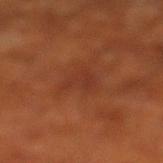The lesion was tiled from a total-body skin photograph and was not biopsied.
Longest diameter approximately 3 mm.
The lesion is on the left lower leg.
A male subject, approximately 70 years of age.
This image is a 15 mm lesion crop taken from a total-body photograph.